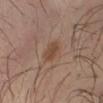biopsy status: total-body-photography surveillance lesion; no biopsy | illumination: cross-polarized | TBP lesion metrics: a lesion area of about 4 mm², an outline eccentricity of about 0.65 (0 = round, 1 = elongated), and a symmetry-axis asymmetry near 0.2; a border-irregularity index near 2/10, a within-lesion color-variation index near 2/10, and a peripheral color-asymmetry measure near 0.5; a nevus-likeness score of about 75/100 | body site: the left forearm | image source: ~15 mm tile from a whole-body skin photo | patient: male, aged approximately 40.